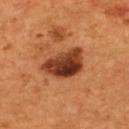Q: Was this lesion biopsied?
A: catalogued during a skin exam; not biopsied
Q: Who is the patient?
A: male, roughly 55 years of age
Q: What kind of image is this?
A: 15 mm crop, total-body photography
Q: What is the anatomic site?
A: the upper back
Q: What did automated image analysis measure?
A: a border-irregularity index near 3/10, internal color variation of about 8 on a 0–10 scale, and radial color variation of about 3; a classifier nevus-likeness of about 75/100 and a detector confidence of about 100 out of 100 that the crop contains a lesion